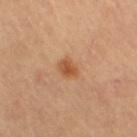biopsy_status: not biopsied; imaged during a skin examination
lighting: cross-polarized
image:
  source: total-body photography crop
  field_of_view_mm: 15
automated_metrics:
  eccentricity: 0.7
  cielab_L: 55
  cielab_a: 25
  cielab_b: 38
  vs_skin_darker_L: 10.0
  vs_skin_contrast_norm: 7.5
  nevus_likeness_0_100: 95
  lesion_detection_confidence_0_100: 100
patient:
  sex: female
  age_approx: 60
lesion_size:
  long_diameter_mm_approx: 3.0
site: leg